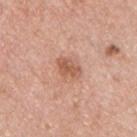<case>
<biopsy_status>not biopsied; imaged during a skin examination</biopsy_status>
<automated_metrics>
  <area_mm2_approx>5.5</area_mm2_approx>
  <eccentricity>0.75</eccentricity>
  <shape_asymmetry>0.25</shape_asymmetry>
  <cielab_L>58</cielab_L>
  <cielab_a>24</cielab_a>
  <cielab_b>31</cielab_b>
  <vs_skin_darker_L>10.0</vs_skin_darker_L>
  <vs_skin_contrast_norm>7.0</vs_skin_contrast_norm>
  <color_variation_0_10>2.5</color_variation_0_10>
  <peripheral_color_asymmetry>1.0</peripheral_color_asymmetry>
  <nevus_likeness_0_100>20</nevus_likeness_0_100>
  <lesion_detection_confidence_0_100>100</lesion_detection_confidence_0_100>
</automated_metrics>
<patient>
  <sex>male</sex>
  <age_approx>40</age_approx>
</patient>
<image>
  <source>total-body photography crop</source>
  <field_of_view_mm>15</field_of_view_mm>
</image>
<site>chest</site>
<lighting>white-light</lighting>
<lesion_size>
  <long_diameter_mm_approx>3.0</long_diameter_mm_approx>
</lesion_size>
</case>